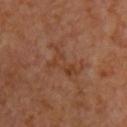Notes:
* workup · catalogued during a skin exam; not biopsied
* diameter · about 5.5 mm
* patient · male, aged 58–62
* imaging modality · total-body-photography crop, ~15 mm field of view
* tile lighting · cross-polarized illumination
* site · the front of the torso